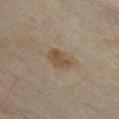No biopsy was performed on this lesion — it was imaged during a full skin examination and was not determined to be concerning. The lesion's longest dimension is about 3.5 mm. A female patient aged approximately 35. The lesion is located on the chest. A 15 mm close-up tile from a total-body photography series done for melanoma screening. This is a cross-polarized tile. Automated tile analysis of the lesion measured a footprint of about 7 mm², an eccentricity of roughly 0.75, and a shape-asymmetry score of about 0.2 (0 = symmetric). And it measured border irregularity of about 2 on a 0–10 scale and a within-lesion color-variation index near 2.5/10. It also reported a detector confidence of about 100 out of 100 that the crop contains a lesion.